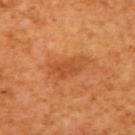Imaged during a routine full-body skin examination; the lesion was not biopsied and no histopathology is available. On the right upper arm. Longest diameter approximately 5 mm. A 15 mm close-up extracted from a 3D total-body photography capture. This is a cross-polarized tile. A female patient approximately 55 years of age.